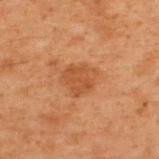The lesion's longest dimension is about 3.5 mm.
The lesion is on the upper back.
Captured under cross-polarized illumination.
A male subject, approximately 70 years of age.
Automated tile analysis of the lesion measured an area of roughly 8.5 mm², an outline eccentricity of about 0.35 (0 = round, 1 = elongated), and two-axis asymmetry of about 0.2. And it measured roughly 7 lightness units darker than nearby skin and a lesion-to-skin contrast of about 6 (normalized; higher = more distinct).
A region of skin cropped from a whole-body photographic capture, roughly 15 mm wide.